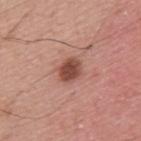biopsy status: total-body-photography surveillance lesion; no biopsy
lesion diameter: ~3 mm (longest diameter)
lighting: white-light
imaging modality: ~15 mm crop, total-body skin-cancer survey
patient: male, in their mid-50s